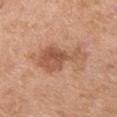<tbp_lesion>
  <biopsy_status>not biopsied; imaged during a skin examination</biopsy_status>
  <site>chest</site>
  <automated_metrics>
    <eccentricity>0.9</eccentricity>
    <shape_asymmetry>0.5</shape_asymmetry>
    <nevus_likeness_0_100>30</nevus_likeness_0_100>
    <lesion_detection_confidence_0_100>100</lesion_detection_confidence_0_100>
  </automated_metrics>
  <patient>
    <sex>male</sex>
    <age_approx>65</age_approx>
  </patient>
  <image>
    <source>total-body photography crop</source>
    <field_of_view_mm>15</field_of_view_mm>
  </image>
</tbp_lesion>